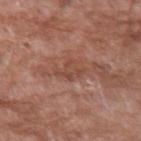lesion diameter: ≈3 mm | patient: male, aged 58 to 62 | TBP lesion metrics: a footprint of about 5.5 mm², an outline eccentricity of about 0.7 (0 = round, 1 = elongated), and a shape-asymmetry score of about 0.3 (0 = symmetric); a lesion–skin lightness drop of about 7 and a lesion-to-skin contrast of about 5.5 (normalized; higher = more distinct) | image source: 15 mm crop, total-body photography | site: the left upper arm.